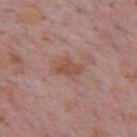biopsy_status: not biopsied; imaged during a skin examination
patient:
  sex: male
  age_approx: 75
image:
  source: total-body photography crop
  field_of_view_mm: 15
site: mid back
lesion_size:
  long_diameter_mm_approx: 3.5